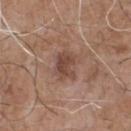follow-up: no biopsy performed (imaged during a skin exam)
size: ≈3.5 mm
image source: ~15 mm tile from a whole-body skin photo
image-analysis metrics: an area of roughly 6.5 mm² and an outline eccentricity of about 0.7 (0 = round, 1 = elongated); a lesion color around L≈45 a*≈20 b*≈25 in CIELAB and a lesion–skin lightness drop of about 10
subject: male, aged 68–72
lighting: white-light illumination
body site: the front of the torso